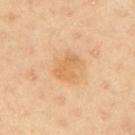Assessment: The lesion was tiled from a total-body skin photograph and was not biopsied. Background: Cropped from a total-body skin-imaging series; the visible field is about 15 mm. Imaged with cross-polarized lighting. A male patient in their 40s. The recorded lesion diameter is about 3.5 mm. Located on the right upper arm.